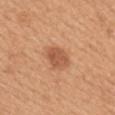workup: catalogued during a skin exam; not biopsied
illumination: white-light illumination
size: ≈3 mm
imaging modality: total-body-photography crop, ~15 mm field of view
image-analysis metrics: a lesion area of about 6.5 mm², a shape eccentricity near 0.6, and a shape-asymmetry score of about 0.2 (0 = symmetric); border irregularity of about 1.5 on a 0–10 scale, a color-variation rating of about 2.5/10, and a peripheral color-asymmetry measure near 1; an automated nevus-likeness rating near 90 out of 100 and a detector confidence of about 100 out of 100 that the crop contains a lesion
subject: female, aged around 50
body site: the mid back